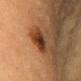{"biopsy_status": "not biopsied; imaged during a skin examination", "site": "right upper arm", "patient": {"sex": "female", "age_approx": 65}, "image": {"source": "total-body photography crop", "field_of_view_mm": 15}}